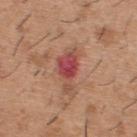Clinical impression:
Captured during whole-body skin photography for melanoma surveillance; the lesion was not biopsied.
Clinical summary:
The lesion is located on the upper back. The recorded lesion diameter is about 5 mm. The lesion-visualizer software estimated an area of roughly 8 mm², a shape eccentricity near 0.85, and two-axis asymmetry of about 0.5. And it measured an average lesion color of about L≈48 a*≈31 b*≈26 (CIELAB) and a lesion–skin lightness drop of about 12. And it measured a border-irregularity index near 5.5/10, a within-lesion color-variation index near 4.5/10, and radial color variation of about 1. The analysis additionally found a nevus-likeness score of about 0/100 and a lesion-detection confidence of about 100/100. A roughly 15 mm field-of-view crop from a total-body skin photograph. Captured under white-light illumination. A male patient, aged 38 to 42.